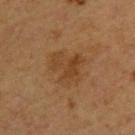<tbp_lesion>
  <biopsy_status>not biopsied; imaged during a skin examination</biopsy_status>
  <patient>
    <sex>male</sex>
    <age_approx>60</age_approx>
  </patient>
  <lesion_size>
    <long_diameter_mm_approx>4.0</long_diameter_mm_approx>
  </lesion_size>
  <image>
    <source>total-body photography crop</source>
    <field_of_view_mm>15</field_of_view_mm>
  </image>
  <site>upper back</site>
</tbp_lesion>